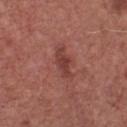No biopsy was performed on this lesion — it was imaged during a full skin examination and was not determined to be concerning. The patient is a male roughly 75 years of age. Cropped from a total-body skin-imaging series; the visible field is about 15 mm. Captured under white-light illumination. The lesion is located on the chest. The recorded lesion diameter is about 4 mm.